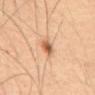Assessment:
No biopsy was performed on this lesion — it was imaged during a full skin examination and was not determined to be concerning.
Image and clinical context:
The lesion-visualizer software estimated an area of roughly 4 mm², a shape eccentricity near 0.55, and two-axis asymmetry of about 0.15. It also reported a mean CIELAB color near L≈57 a*≈22 b*≈34 and a normalized lesion–skin contrast near 8.5. The analysis additionally found a within-lesion color-variation index near 6/10 and radial color variation of about 2. This is a cross-polarized tile. Located on the abdomen. The lesion's longest dimension is about 2.5 mm. A 15 mm close-up tile from a total-body photography series done for melanoma screening. A male patient aged 48 to 52.